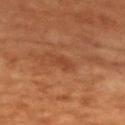Notes:
• follow-up: no biopsy performed (imaged during a skin exam)
• subject: female, aged around 80
• location: the upper back
• image: total-body-photography crop, ~15 mm field of view
• size: ~2.5 mm (longest diameter)